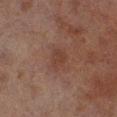Notes:
* lesion diameter · about 2.5 mm
* body site · the leg
* illumination · cross-polarized illumination
* imaging modality · ~15 mm crop, total-body skin-cancer survey
* patient · male, aged 68 to 72
* automated metrics · a footprint of about 4.5 mm² and two-axis asymmetry of about 0.3; a mean CIELAB color near L≈31 a*≈17 b*≈22, roughly 5 lightness units darker than nearby skin, and a normalized lesion–skin contrast near 5; border irregularity of about 2.5 on a 0–10 scale, internal color variation of about 1 on a 0–10 scale, and a peripheral color-asymmetry measure near 0.5